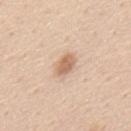{"biopsy_status": "not biopsied; imaged during a skin examination", "site": "mid back", "image": {"source": "total-body photography crop", "field_of_view_mm": 15}, "lighting": "white-light", "patient": {"sex": "male", "age_approx": 45}}